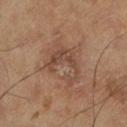* notes — imaged on a skin check; not biopsied
* acquisition — ~15 mm tile from a whole-body skin photo
* site — the left lower leg
* subject — male, aged 63–67